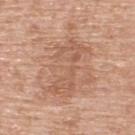The lesion was photographed on a routine skin check and not biopsied; there is no pathology result. This image is a 15 mm lesion crop taken from a total-body photograph. Measured at roughly 7 mm in maximum diameter. An algorithmic analysis of the crop reported an area of roughly 16 mm² and a symmetry-axis asymmetry near 0.45. The analysis additionally found a color-variation rating of about 3/10. A male patient about 60 years old. The lesion is on the upper back.